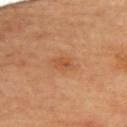Q: Was a biopsy performed?
A: imaged on a skin check; not biopsied
Q: How was this image acquired?
A: 15 mm crop, total-body photography
Q: Lesion location?
A: the upper back
Q: Lesion size?
A: about 2.5 mm
Q: What are the patient's age and sex?
A: female, aged 58–62
Q: What did automated image analysis measure?
A: a classifier nevus-likeness of about 15/100
Q: What lighting was used for the tile?
A: cross-polarized illumination